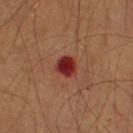The lesion was tiled from a total-body skin photograph and was not biopsied.
The lesion is on the upper back.
Automated tile analysis of the lesion measured a lesion area of about 5 mm², an eccentricity of roughly 0.2, and a symmetry-axis asymmetry near 0.25. And it measured a border-irregularity rating of about 2/10, internal color variation of about 3.5 on a 0–10 scale, and peripheral color asymmetry of about 1.
The patient is a male in their 70s.
Longest diameter approximately 2.5 mm.
Cropped from a whole-body photographic skin survey; the tile spans about 15 mm.
The tile uses cross-polarized illumination.